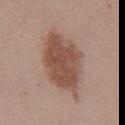biopsy status = imaged on a skin check; not biopsied
patient = male, aged 38–42
illumination = white-light
image-analysis metrics = two-axis asymmetry of about 0.15; a lesion color around L≈50 a*≈19 b*≈26 in CIELAB, roughly 12 lightness units darker than nearby skin, and a normalized border contrast of about 8.5; border irregularity of about 2.5 on a 0–10 scale, internal color variation of about 3.5 on a 0–10 scale, and radial color variation of about 1; a classifier nevus-likeness of about 90/100 and a lesion-detection confidence of about 100/100
location = the abdomen
acquisition = ~15 mm tile from a whole-body skin photo